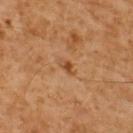Recorded during total-body skin imaging; not selected for excision or biopsy. Measured at roughly 2.5 mm in maximum diameter. Imaged with cross-polarized lighting. A male patient about 60 years old. The lesion is on the upper back. The total-body-photography lesion software estimated an area of roughly 3 mm², an eccentricity of roughly 0.85, and a shape-asymmetry score of about 0.35 (0 = symmetric). The analysis additionally found a lesion color around L≈45 a*≈21 b*≈35 in CIELAB and a normalized lesion–skin contrast near 6.5. Cropped from a whole-body photographic skin survey; the tile spans about 15 mm.